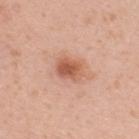notes = catalogued during a skin exam; not biopsied | tile lighting = white-light | site = the back | automated lesion analysis = a nevus-likeness score of about 85/100 and lesion-presence confidence of about 100/100 | patient = female, aged approximately 30 | diameter = about 3 mm | image = ~15 mm crop, total-body skin-cancer survey.